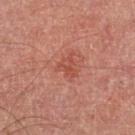This lesion was catalogued during total-body skin photography and was not selected for biopsy. On the left lower leg. Imaged with cross-polarized lighting. A male subject aged approximately 70. Cropped from a whole-body photographic skin survey; the tile spans about 15 mm. The lesion-visualizer software estimated a lesion area of about 3.5 mm², an outline eccentricity of about 0.6 (0 = round, 1 = elongated), and two-axis asymmetry of about 0.5. And it measured roughly 7 lightness units darker than nearby skin and a normalized lesion–skin contrast near 5.5. It also reported internal color variation of about 3.5 on a 0–10 scale and peripheral color asymmetry of about 1.